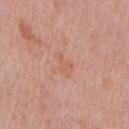{"biopsy_status": "not biopsied; imaged during a skin examination", "patient": {"sex": "male", "age_approx": 70}, "lesion_size": {"long_diameter_mm_approx": 2.5}, "site": "chest", "image": {"source": "total-body photography crop", "field_of_view_mm": 15}}